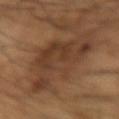workup — imaged on a skin check; not biopsied | patient — male, aged 63 to 67 | imaging modality — ~15 mm crop, total-body skin-cancer survey | lesion diameter — ≈7.5 mm | body site — the right forearm.